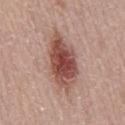Q: Is there a histopathology result?
A: no biopsy performed (imaged during a skin exam)
Q: Lesion location?
A: the mid back
Q: How was this image acquired?
A: 15 mm crop, total-body photography
Q: Patient demographics?
A: female, about 60 years old